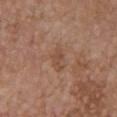Assessment: This lesion was catalogued during total-body skin photography and was not selected for biopsy. Image and clinical context: About 2.5 mm across. Captured under white-light illumination. A female subject, roughly 65 years of age. From the chest. An algorithmic analysis of the crop reported a mean CIELAB color near L≈47 a*≈20 b*≈29, a lesion–skin lightness drop of about 7, and a normalized lesion–skin contrast near 5. It also reported border irregularity of about 5.5 on a 0–10 scale, a color-variation rating of about 0/10, and peripheral color asymmetry of about 0. It also reported an automated nevus-likeness rating near 0 out of 100 and a lesion-detection confidence of about 100/100. A 15 mm crop from a total-body photograph taken for skin-cancer surveillance.